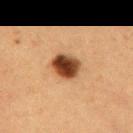{
  "biopsy_status": "not biopsied; imaged during a skin examination",
  "patient": {
    "sex": "female",
    "age_approx": 40
  },
  "image": {
    "source": "total-body photography crop",
    "field_of_view_mm": 15
  },
  "automated_metrics": {
    "area_mm2_approx": 9.0,
    "eccentricity": 0.65,
    "cielab_L": 38,
    "cielab_a": 20,
    "cielab_b": 31,
    "vs_skin_contrast_norm": 14.0,
    "nevus_likeness_0_100": 100
  },
  "lighting": "cross-polarized",
  "site": "mid back"
}